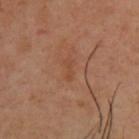<record>
  <patient>
    <sex>male</sex>
    <age_approx>40</age_approx>
  </patient>
  <site>upper back</site>
  <lighting>cross-polarized</lighting>
  <image>
    <source>total-body photography crop</source>
    <field_of_view_mm>15</field_of_view_mm>
  </image>
  <lesion_size>
    <long_diameter_mm_approx>2.5</long_diameter_mm_approx>
  </lesion_size>
</record>